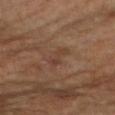Part of a total-body skin-imaging series; this lesion was reviewed on a skin check and was not flagged for biopsy.